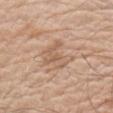No biopsy was performed on this lesion — it was imaged during a full skin examination and was not determined to be concerning. The patient is a male about 65 years old. The total-body-photography lesion software estimated a lesion area of about 9 mm², an eccentricity of roughly 0.35, and two-axis asymmetry of about 0.5. The software also gave a mean CIELAB color near L≈60 a*≈18 b*≈31, a lesion–skin lightness drop of about 8, and a normalized border contrast of about 5. And it measured a color-variation rating of about 3.5/10. And it measured an automated nevus-likeness rating near 0 out of 100 and a lesion-detection confidence of about 90/100. The lesion's longest dimension is about 4 mm. This image is a 15 mm lesion crop taken from a total-body photograph. Located on the right upper arm. This is a white-light tile.